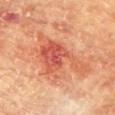Captured during whole-body skin photography for melanoma surveillance; the lesion was not biopsied.
Imaged with cross-polarized lighting.
Approximately 8.5 mm at its widest.
Located on the front of the torso.
A 15 mm close-up extracted from a 3D total-body photography capture.
A female patient aged around 80.